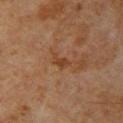Clinical impression: The lesion was photographed on a routine skin check and not biopsied; there is no pathology result. Image and clinical context: This image is a 15 mm lesion crop taken from a total-body photograph. The subject is a male approximately 60 years of age. The lesion is on the upper back.